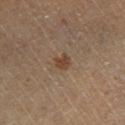Q: Was a biopsy performed?
A: total-body-photography surveillance lesion; no biopsy
Q: How was this image acquired?
A: ~15 mm tile from a whole-body skin photo
Q: What are the patient's age and sex?
A: male, roughly 60 years of age
Q: Where on the body is the lesion?
A: the right lower leg